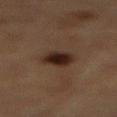biopsy_status: not biopsied; imaged during a skin examination
lighting: cross-polarized
patient:
  sex: male
  age_approx: 85+
automated_metrics:
  area_mm2_approx: 7.5
  eccentricity: 0.65
  cielab_L: 21
  cielab_a: 14
  cielab_b: 19
image:
  source: total-body photography crop
  field_of_view_mm: 15
site: mid back